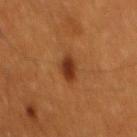Part of a total-body skin-imaging series; this lesion was reviewed on a skin check and was not flagged for biopsy. The tile uses cross-polarized illumination. The lesion's longest dimension is about 3.5 mm. This image is a 15 mm lesion crop taken from a total-body photograph. From the back. A male patient, aged approximately 60.